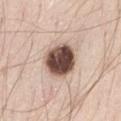This is a white-light tile.
A 15 mm close-up tile from a total-body photography series done for melanoma screening.
A male subject aged 38–42.
The lesion-visualizer software estimated a lesion area of about 15 mm², an outline eccentricity of about 0.4 (0 = round, 1 = elongated), and a shape-asymmetry score of about 0.15 (0 = symmetric). It also reported a border-irregularity rating of about 1.5/10, a color-variation rating of about 7.5/10, and a peripheral color-asymmetry measure near 2.5. The software also gave a lesion-detection confidence of about 100/100.
On the abdomen.